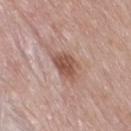follow-up: imaged on a skin check; not biopsied
acquisition: ~15 mm tile from a whole-body skin photo
site: the right thigh
subject: female, approximately 60 years of age
lighting: white-light
image-analysis metrics: an area of roughly 7.5 mm², an outline eccentricity of about 0.75 (0 = round, 1 = elongated), and two-axis asymmetry of about 0.3; border irregularity of about 3 on a 0–10 scale, a within-lesion color-variation index near 2.5/10, and radial color variation of about 1; a classifier nevus-likeness of about 85/100 and a lesion-detection confidence of about 100/100
lesion size: about 4 mm